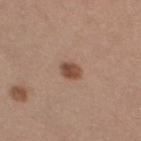Clinical impression: No biopsy was performed on this lesion — it was imaged during a full skin examination and was not determined to be concerning. Clinical summary: Cropped from a whole-body photographic skin survey; the tile spans about 15 mm. Automated image analysis of the tile measured a lesion color around L≈47 a*≈20 b*≈28 in CIELAB and a normalized lesion–skin contrast near 9.5. It also reported a border-irregularity rating of about 1.5/10, a within-lesion color-variation index near 3.5/10, and radial color variation of about 1.5. And it measured a nevus-likeness score of about 95/100. On the right thigh. A female patient in their mid- to late 30s. The tile uses white-light illumination. Measured at roughly 2.5 mm in maximum diameter.